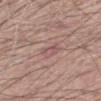| feature | finding |
|---|---|
| follow-up | catalogued during a skin exam; not biopsied |
| subject | male, aged 58–62 |
| acquisition | total-body-photography crop, ~15 mm field of view |
| anatomic site | the mid back |